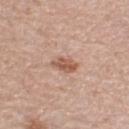notes: no biopsy performed (imaged during a skin exam)
anatomic site: the right lower leg
patient: female, about 65 years old
image: ~15 mm tile from a whole-body skin photo
illumination: white-light illumination
lesion size: ~3 mm (longest diameter)